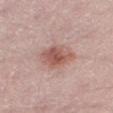  biopsy_status: not biopsied; imaged during a skin examination
  image:
    source: total-body photography crop
    field_of_view_mm: 15
  patient:
    sex: male
    age_approx: 50
  site: right thigh
  lesion_size:
    long_diameter_mm_approx: 4.5
  automated_metrics:
    border_irregularity_0_10: 2.0
    color_variation_0_10: 4.5
    peripheral_color_asymmetry: 1.0